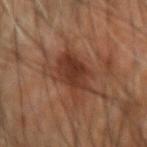Acquisition and patient details:
A 15 mm crop from a total-body photograph taken for skin-cancer surveillance. Imaged with cross-polarized lighting. The recorded lesion diameter is about 4.5 mm. From the right upper arm. A male patient, in their 60s.